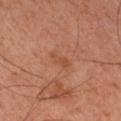No biopsy was performed on this lesion — it was imaged during a full skin examination and was not determined to be concerning. The lesion is on the right upper arm. A lesion tile, about 15 mm wide, cut from a 3D total-body photograph. The subject is a male aged 43–47.